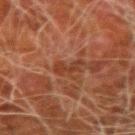Q: Is there a histopathology result?
A: catalogued during a skin exam; not biopsied
Q: Lesion location?
A: the left forearm
Q: Patient demographics?
A: male, aged 78–82
Q: What kind of image is this?
A: ~15 mm tile from a whole-body skin photo
Q: Illumination type?
A: cross-polarized illumination